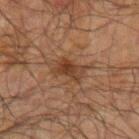Impression: Imaged during a routine full-body skin examination; the lesion was not biopsied and no histopathology is available. Image and clinical context: A region of skin cropped from a whole-body photographic capture, roughly 15 mm wide. The lesion's longest dimension is about 3.5 mm. Located on the arm. A male patient in their 70s. Captured under cross-polarized illumination. An algorithmic analysis of the crop reported an average lesion color of about L≈32 a*≈17 b*≈26 (CIELAB) and a lesion-to-skin contrast of about 7.5 (normalized; higher = more distinct). The analysis additionally found a classifier nevus-likeness of about 65/100 and a detector confidence of about 100 out of 100 that the crop contains a lesion.